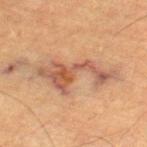location = the right thigh | image = ~15 mm tile from a whole-body skin photo | subject = male, aged 83 or over.